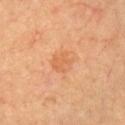<tbp_lesion>
  <biopsy_status>not biopsied; imaged during a skin examination</biopsy_status>
  <site>chest</site>
  <patient>
    <sex>male</sex>
    <age_approx>70</age_approx>
  </patient>
  <lighting>cross-polarized</lighting>
  <lesion_size>
    <long_diameter_mm_approx>2.5</long_diameter_mm_approx>
  </lesion_size>
  <image>
    <source>total-body photography crop</source>
    <field_of_view_mm>15</field_of_view_mm>
  </image>
</tbp_lesion>